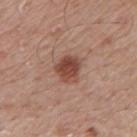patient: male, aged around 75; lesion size: about 3.5 mm; location: the mid back; image source: ~15 mm tile from a whole-body skin photo; automated lesion analysis: border irregularity of about 2.5 on a 0–10 scale, internal color variation of about 4 on a 0–10 scale, and radial color variation of about 1.5; lighting: white-light illumination.